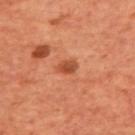Recorded during total-body skin imaging; not selected for excision or biopsy. The tile uses cross-polarized illumination. The recorded lesion diameter is about 2.5 mm. A 15 mm close-up extracted from a 3D total-body photography capture. The patient is a male in their 70s. Located on the upper back.